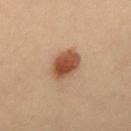notes = catalogued during a skin exam; not biopsied
lighting = cross-polarized
location = the back
subject = female, aged approximately 30
imaging modality = ~15 mm crop, total-body skin-cancer survey